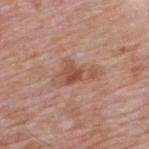The subject is a male about 60 years old. Longest diameter approximately 4.5 mm. This image is a 15 mm lesion crop taken from a total-body photograph. Captured under white-light illumination. The lesion-visualizer software estimated an area of roughly 7.5 mm², a shape eccentricity near 0.9, and a shape-asymmetry score of about 0.5 (0 = symmetric). On the back.